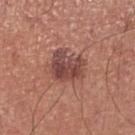biopsy status: total-body-photography surveillance lesion; no biopsy
anatomic site: the right lower leg
imaging modality: total-body-photography crop, ~15 mm field of view
subject: male, in their 70s
tile lighting: white-light
lesion size: about 4 mm